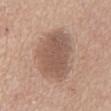The lesion was photographed on a routine skin check and not biopsied; there is no pathology result.
The lesion is on the abdomen.
A female patient, aged 53 to 57.
The lesion's longest dimension is about 7.5 mm.
A roughly 15 mm field-of-view crop from a total-body skin photograph.